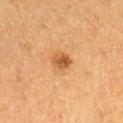The lesion was tiled from a total-body skin photograph and was not biopsied. The lesion is located on the right lower leg. A lesion tile, about 15 mm wide, cut from a 3D total-body photograph. The subject is a female aged around 40.